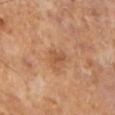| key | value |
|---|---|
| biopsy status | imaged on a skin check; not biopsied |
| patient | male, roughly 65 years of age |
| image | ~15 mm tile from a whole-body skin photo |